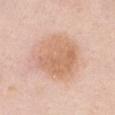Clinical impression: Captured during whole-body skin photography for melanoma surveillance; the lesion was not biopsied. Context: A close-up tile cropped from a whole-body skin photograph, about 15 mm across. A female patient, in their mid-60s. The lesion-visualizer software estimated about 9 CIELAB-L* units darker than the surrounding skin. Located on the chest. Captured under white-light illumination. The recorded lesion diameter is about 6.5 mm.